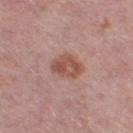The lesion was photographed on a routine skin check and not biopsied; there is no pathology result.